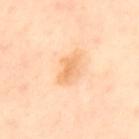<tbp_lesion>
<biopsy_status>not biopsied; imaged during a skin examination</biopsy_status>
<automated_metrics>
  <area_mm2_approx>5.0</area_mm2_approx>
  <eccentricity>0.9</eccentricity>
  <shape_asymmetry>0.35</shape_asymmetry>
  <border_irregularity_0_10>4.0</border_irregularity_0_10>
  <peripheral_color_asymmetry>0.5</peripheral_color_asymmetry>
  <nevus_likeness_0_100>5</nevus_likeness_0_100>
</automated_metrics>
<lighting>cross-polarized</lighting>
<patient>
  <sex>female</sex>
  <age_approx>55</age_approx>
</patient>
<lesion_size>
  <long_diameter_mm_approx>3.5</long_diameter_mm_approx>
</lesion_size>
<image>
  <source>total-body photography crop</source>
  <field_of_view_mm>15</field_of_view_mm>
</image>
<site>back</site>
</tbp_lesion>